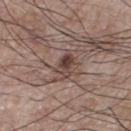Imaged during a routine full-body skin examination; the lesion was not biopsied and no histopathology is available. On the chest. A male subject in their mid-70s. About 3 mm across. Imaged with white-light lighting. A 15 mm close-up tile from a total-body photography series done for melanoma screening.